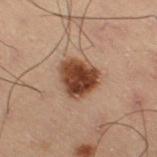No biopsy was performed on this lesion — it was imaged during a full skin examination and was not determined to be concerning.
From the right thigh.
A male subject, in their mid- to late 50s.
A close-up tile cropped from a whole-body skin photograph, about 15 mm across.
The tile uses cross-polarized illumination.
An algorithmic analysis of the crop reported a lesion area of about 13 mm² and two-axis asymmetry of about 0.25. It also reported an average lesion color of about L≈34 a*≈18 b*≈25 (CIELAB) and a lesion-to-skin contrast of about 13 (normalized; higher = more distinct). It also reported border irregularity of about 2 on a 0–10 scale and peripheral color asymmetry of about 1.5. The analysis additionally found a nevus-likeness score of about 100/100.
Longest diameter approximately 4.5 mm.